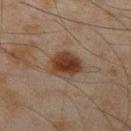| field | value |
|---|---|
| notes | catalogued during a skin exam; not biopsied |
| patient | male, in their mid-40s |
| anatomic site | the left thigh |
| lighting | cross-polarized |
| lesion diameter | about 4 mm |
| TBP lesion metrics | an eccentricity of roughly 0.5 and a shape-asymmetry score of about 0.2 (0 = symmetric); an average lesion color of about L≈30 a*≈16 b*≈24 (CIELAB), about 11 CIELAB-L* units darker than the surrounding skin, and a normalized border contrast of about 11; a border-irregularity rating of about 2/10, a within-lesion color-variation index near 4.5/10, and peripheral color asymmetry of about 1.5; an automated nevus-likeness rating near 100 out of 100 and a lesion-detection confidence of about 100/100 |
| acquisition | total-body-photography crop, ~15 mm field of view |